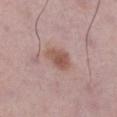{"biopsy_status": "not biopsied; imaged during a skin examination", "site": "left lower leg", "lesion_size": {"long_diameter_mm_approx": 3.5}, "image": {"source": "total-body photography crop", "field_of_view_mm": 15}, "automated_metrics": {"eccentricity": 0.6, "shape_asymmetry": 0.25, "cielab_L": 54, "cielab_a": 20, "cielab_b": 25, "vs_skin_darker_L": 10.0, "vs_skin_contrast_norm": 7.5, "border_irregularity_0_10": 2.5}, "patient": {"sex": "male", "age_approx": 50}, "lighting": "white-light"}